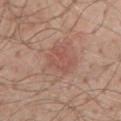The subject is a male aged 48 to 52. Automated image analysis of the tile measured a lesion color around L≈54 a*≈21 b*≈26 in CIELAB, about 6 CIELAB-L* units darker than the surrounding skin, and a normalized lesion–skin contrast near 4.5. A 15 mm crop from a total-body photograph taken for skin-cancer surveillance. The lesion is located on the chest. This is a white-light tile. Measured at roughly 6 mm in maximum diameter.